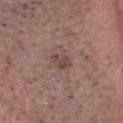  biopsy_status: not biopsied; imaged during a skin examination
  patient:
    sex: male
    age_approx: 55
  image:
    source: total-body photography crop
    field_of_view_mm: 15
  lighting: white-light
  automated_metrics:
    shape_asymmetry: 0.5
    vs_skin_darker_L: 8.0
    vs_skin_contrast_norm: 6.5
    border_irregularity_0_10: 5.0
    color_variation_0_10: 1.0
    peripheral_color_asymmetry: 0.5
    nevus_likeness_0_100: 0
    lesion_detection_confidence_0_100: 100
  lesion_size:
    long_diameter_mm_approx: 2.5